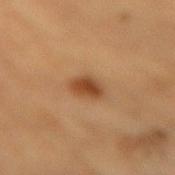Impression:
No biopsy was performed on this lesion — it was imaged during a full skin examination and was not determined to be concerning.
Image and clinical context:
The lesion is on the mid back. The subject is a male in their mid- to late 80s or older. The recorded lesion diameter is about 3 mm. A 15 mm close-up extracted from a 3D total-body photography capture.